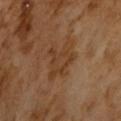This lesion was catalogued during total-body skin photography and was not selected for biopsy. A 15 mm crop from a total-body photograph taken for skin-cancer surveillance. The tile uses cross-polarized illumination. A male subject aged around 65. The recorded lesion diameter is about 4 mm.